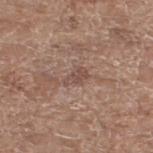Q: Was a biopsy performed?
A: imaged on a skin check; not biopsied
Q: What are the patient's age and sex?
A: female, approximately 75 years of age
Q: What kind of image is this?
A: ~15 mm tile from a whole-body skin photo
Q: What is the anatomic site?
A: the right thigh
Q: What is the lesion's diameter?
A: about 3 mm
Q: Automated lesion metrics?
A: an outline eccentricity of about 0.8 (0 = round, 1 = elongated); a lesion color around L≈49 a*≈18 b*≈24 in CIELAB, roughly 7 lightness units darker than nearby skin, and a lesion-to-skin contrast of about 5.5 (normalized; higher = more distinct); a border-irregularity index near 4/10, internal color variation of about 1 on a 0–10 scale, and radial color variation of about 0.5; a nevus-likeness score of about 0/100 and lesion-presence confidence of about 95/100